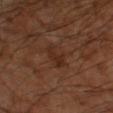Captured during whole-body skin photography for melanoma surveillance; the lesion was not biopsied. A male subject, in their mid-50s. Captured under cross-polarized illumination. About 3.5 mm across. The lesion is on the left upper arm. A roughly 15 mm field-of-view crop from a total-body skin photograph.